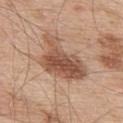The lesion was photographed on a routine skin check and not biopsied; there is no pathology result. A male patient, approximately 55 years of age. A roughly 15 mm field-of-view crop from a total-body skin photograph. The tile uses white-light illumination. Measured at roughly 7 mm in maximum diameter. Automated image analysis of the tile measured a footprint of about 20 mm², an outline eccentricity of about 0.75 (0 = round, 1 = elongated), and a shape-asymmetry score of about 0.5 (0 = symmetric). The software also gave an average lesion color of about L≈53 a*≈21 b*≈30 (CIELAB), roughly 13 lightness units darker than nearby skin, and a normalized border contrast of about 8.5. The software also gave a border-irregularity index near 5/10 and a peripheral color-asymmetry measure near 2. On the upper back.